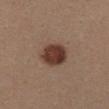| field | value |
|---|---|
| biopsy status | catalogued during a skin exam; not biopsied |
| image-analysis metrics | an average lesion color of about L≈38 a*≈19 b*≈25 (CIELAB) and a normalized border contrast of about 11.5 |
| lesion size | ~3.5 mm (longest diameter) |
| illumination | white-light |
| patient | female, in their 30s |
| site | the chest |
| acquisition | ~15 mm crop, total-body skin-cancer survey |